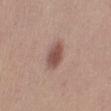Assessment:
Recorded during total-body skin imaging; not selected for excision or biopsy.